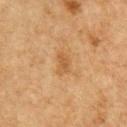Assessment: Recorded during total-body skin imaging; not selected for excision or biopsy. Image and clinical context: The tile uses cross-polarized illumination. Longest diameter approximately 2.5 mm. The lesion-visualizer software estimated border irregularity of about 2.5 on a 0–10 scale, a within-lesion color-variation index near 1.5/10, and peripheral color asymmetry of about 0.5. The patient is a male in their mid- to late 70s. A lesion tile, about 15 mm wide, cut from a 3D total-body photograph. On the chest.